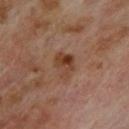Captured during whole-body skin photography for melanoma surveillance; the lesion was not biopsied. On the upper back. About 3.5 mm across. Imaged with cross-polarized lighting. The subject is a male aged 68 to 72. This image is a 15 mm lesion crop taken from a total-body photograph.